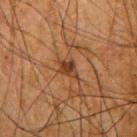The lesion was photographed on a routine skin check and not biopsied; there is no pathology result. This image is a 15 mm lesion crop taken from a total-body photograph. A male patient aged approximately 65. The lesion's longest dimension is about 3.5 mm. This is a cross-polarized tile. The lesion is on the right upper arm.